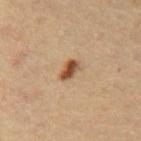Captured during whole-body skin photography for melanoma surveillance; the lesion was not biopsied.